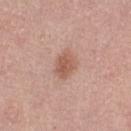Q: Is there a histopathology result?
A: catalogued during a skin exam; not biopsied
Q: What are the patient's age and sex?
A: female, aged 63–67
Q: How was this image acquired?
A: ~15 mm crop, total-body skin-cancer survey
Q: Lesion location?
A: the right thigh
Q: What lighting was used for the tile?
A: white-light illumination